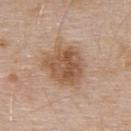follow-up: imaged on a skin check; not biopsied
size: about 5.5 mm
body site: the upper back
image source: total-body-photography crop, ~15 mm field of view
automated metrics: a lesion color around L≈54 a*≈19 b*≈31 in CIELAB, a lesion–skin lightness drop of about 11, and a lesion-to-skin contrast of about 8 (normalized; higher = more distinct); a border-irregularity rating of about 2.5/10, a color-variation rating of about 4.5/10, and radial color variation of about 1.5; a detector confidence of about 100 out of 100 that the crop contains a lesion
tile lighting: white-light illumination
subject: male, roughly 55 years of age